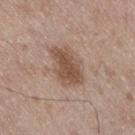notes = catalogued during a skin exam; not biopsied
subject = male, aged 48–52
location = the leg
illumination = white-light
acquisition = ~15 mm crop, total-body skin-cancer survey